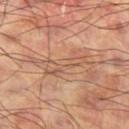Impression: Recorded during total-body skin imaging; not selected for excision or biopsy. Clinical summary: A male patient, about 60 years old. The lesion's longest dimension is about 5 mm. Captured under cross-polarized illumination. A lesion tile, about 15 mm wide, cut from a 3D total-body photograph. The lesion-visualizer software estimated a lesion color around L≈55 a*≈20 b*≈31 in CIELAB, roughly 7 lightness units darker than nearby skin, and a normalized border contrast of about 5. And it measured a border-irregularity rating of about 7/10, internal color variation of about 4 on a 0–10 scale, and peripheral color asymmetry of about 1.5. It also reported a nevus-likeness score of about 0/100 and a lesion-detection confidence of about 55/100. From the left thigh.